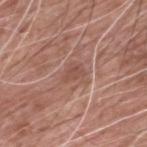Q: Was a biopsy performed?
A: imaged on a skin check; not biopsied
Q: How was this image acquired?
A: ~15 mm crop, total-body skin-cancer survey
Q: Where on the body is the lesion?
A: the back
Q: Who is the patient?
A: male, in their 60s